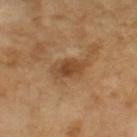  biopsy_status: not biopsied; imaged during a skin examination
  lesion_size:
    long_diameter_mm_approx: 3.5
  image:
    source: total-body photography crop
    field_of_view_mm: 15
  lighting: cross-polarized
  patient:
    sex: male
    age_approx: 65